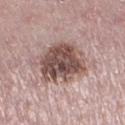- notes — imaged on a skin check; not biopsied
- image source — 15 mm crop, total-body photography
- location — the leg
- subject — female, about 45 years old
- lesion size — about 6 mm
- tile lighting — white-light illumination